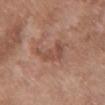Clinical impression:
No biopsy was performed on this lesion — it was imaged during a full skin examination and was not determined to be concerning.
Image and clinical context:
The lesion's longest dimension is about 4.5 mm. This image is a 15 mm lesion crop taken from a total-body photograph. Automated image analysis of the tile measured a footprint of about 7 mm², an outline eccentricity of about 0.85 (0 = round, 1 = elongated), and two-axis asymmetry of about 0.5. The software also gave border irregularity of about 6 on a 0–10 scale and radial color variation of about 1. Imaged with white-light lighting. A female patient in their 70s. The lesion is located on the chest.